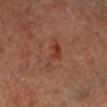Clinical impression:
This lesion was catalogued during total-body skin photography and was not selected for biopsy.
Image and clinical context:
The lesion-visualizer software estimated a footprint of about 7 mm² and a symmetry-axis asymmetry near 0.5. It also reported a lesion color around L≈40 a*≈24 b*≈29 in CIELAB and a lesion–skin lightness drop of about 6. It also reported internal color variation of about 3 on a 0–10 scale and a peripheral color-asymmetry measure near 0.5. Imaged with cross-polarized lighting. The lesion is located on the left lower leg. Cropped from a whole-body photographic skin survey; the tile spans about 15 mm. Measured at roughly 5.5 mm in maximum diameter. A male subject, aged around 65.